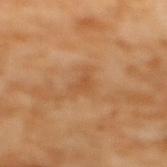Assessment: The lesion was tiled from a total-body skin photograph and was not biopsied. Clinical summary: A female subject aged around 55. A 15 mm crop from a total-body photograph taken for skin-cancer surveillance. Located on the mid back. Approximately 3 mm at its widest.